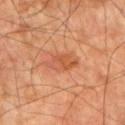Clinical impression:
This lesion was catalogued during total-body skin photography and was not selected for biopsy.
Image and clinical context:
The subject is a male about 70 years old. The lesion is located on the left upper arm. Cropped from a total-body skin-imaging series; the visible field is about 15 mm.